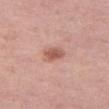Assessment:
The lesion was photographed on a routine skin check and not biopsied; there is no pathology result.
Image and clinical context:
A female subject, in their mid- to late 40s. From the left thigh. Cropped from a whole-body photographic skin survey; the tile spans about 15 mm. This is a white-light tile. Longest diameter approximately 3 mm. The lesion-visualizer software estimated a mean CIELAB color near L≈57 a*≈25 b*≈27, roughly 11 lightness units darker than nearby skin, and a normalized lesion–skin contrast near 7.5. It also reported border irregularity of about 1.5 on a 0–10 scale and a peripheral color-asymmetry measure near 1.